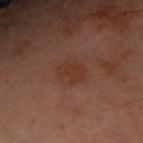Part of a total-body skin-imaging series; this lesion was reviewed on a skin check and was not flagged for biopsy. A region of skin cropped from a whole-body photographic capture, roughly 15 mm wide. A female subject approximately 60 years of age. The lesion is on the front of the torso. This is a cross-polarized tile.